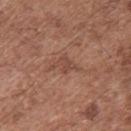Q: Was a biopsy performed?
A: total-body-photography surveillance lesion; no biopsy
Q: Automated lesion metrics?
A: a footprint of about 4 mm² and an outline eccentricity of about 0.8 (0 = round, 1 = elongated); a mean CIELAB color near L≈47 a*≈22 b*≈27, about 7 CIELAB-L* units darker than the surrounding skin, and a normalized border contrast of about 5.5; a classifier nevus-likeness of about 0/100 and lesion-presence confidence of about 100/100
Q: What is the imaging modality?
A: total-body-photography crop, ~15 mm field of view
Q: How was the tile lit?
A: white-light illumination
Q: What are the patient's age and sex?
A: male, about 75 years old
Q: Where on the body is the lesion?
A: the upper back
Q: Lesion size?
A: ~3.5 mm (longest diameter)